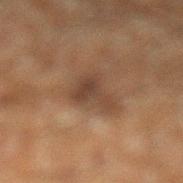Q: Was a biopsy performed?
A: imaged on a skin check; not biopsied
Q: Who is the patient?
A: male, in their 60s
Q: What is the anatomic site?
A: the left lower leg
Q: Automated lesion metrics?
A: an area of roughly 6.5 mm²; a mean CIELAB color near L≈31 a*≈14 b*≈22, a lesion–skin lightness drop of about 7, and a normalized border contrast of about 7.5; a border-irregularity index near 6.5/10; a nevus-likeness score of about 0/100 and lesion-presence confidence of about 100/100
Q: What is the imaging modality?
A: ~15 mm crop, total-body skin-cancer survey
Q: How large is the lesion?
A: ~4 mm (longest diameter)
Q: Illumination type?
A: cross-polarized